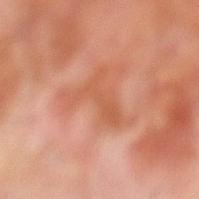Notes:
• follow-up · catalogued during a skin exam; not biopsied
• body site · the leg
• subject · male, aged around 70
• imaging modality · 15 mm crop, total-body photography